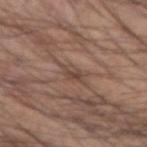Q: Was a biopsy performed?
A: total-body-photography surveillance lesion; no biopsy
Q: What did automated image analysis measure?
A: a border-irregularity index near 4/10, internal color variation of about 2 on a 0–10 scale, and a peripheral color-asymmetry measure near 0.5
Q: What are the patient's age and sex?
A: male, roughly 50 years of age
Q: What lighting was used for the tile?
A: white-light
Q: What is the anatomic site?
A: the arm
Q: What is the lesion's diameter?
A: ~2.5 mm (longest diameter)
Q: How was this image acquired?
A: ~15 mm crop, total-body skin-cancer survey